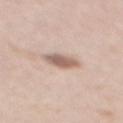• notes — imaged on a skin check; not biopsied
• site — the back
• patient — male, aged approximately 40
• image source — ~15 mm crop, total-body skin-cancer survey